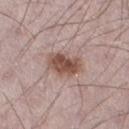biopsy_status: not biopsied; imaged during a skin examination
site: right thigh
lesion_size:
  long_diameter_mm_approx: 3.5
automated_metrics:
  area_mm2_approx: 9.0
  eccentricity: 0.65
  shape_asymmetry: 0.2
  cielab_L: 50
  cielab_a: 19
  cielab_b: 24
  vs_skin_contrast_norm: 9.5
  border_irregularity_0_10: 2.5
  peripheral_color_asymmetry: 1.5
  nevus_likeness_0_100: 90
image:
  source: total-body photography crop
  field_of_view_mm: 15
patient:
  sex: male
  age_approx: 70